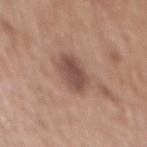biopsy status = total-body-photography surveillance lesion; no biopsy
automated metrics = a footprint of about 9.5 mm² and an eccentricity of roughly 0.75; a lesion-detection confidence of about 100/100
image = 15 mm crop, total-body photography
anatomic site = the mid back
subject = male, aged approximately 70